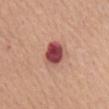Q: Was a biopsy performed?
A: imaged on a skin check; not biopsied
Q: What is the anatomic site?
A: the mid back
Q: What is the imaging modality?
A: ~15 mm tile from a whole-body skin photo
Q: What are the patient's age and sex?
A: male, aged approximately 65